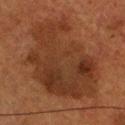Part of a total-body skin-imaging series; this lesion was reviewed on a skin check and was not flagged for biopsy. The recorded lesion diameter is about 12 mm. A 15 mm crop from a total-body photograph taken for skin-cancer surveillance. Captured under cross-polarized illumination. Located on the head or neck. An algorithmic analysis of the crop reported a lesion area of about 60 mm², an outline eccentricity of about 0.8 (0 = round, 1 = elongated), and a shape-asymmetry score of about 0.3 (0 = symmetric). The software also gave a lesion color around L≈27 a*≈18 b*≈25 in CIELAB and roughly 7 lightness units darker than nearby skin. And it measured a border-irregularity index near 5.5/10, a color-variation rating of about 4/10, and radial color variation of about 1.5. A male subject, approximately 60 years of age.